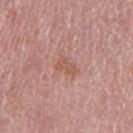Recorded during total-body skin imaging; not selected for excision or biopsy. An algorithmic analysis of the crop reported an average lesion color of about L≈56 a*≈23 b*≈26 (CIELAB), roughly 7 lightness units darker than nearby skin, and a lesion-to-skin contrast of about 5.5 (normalized; higher = more distinct). This is a white-light tile. A female patient, about 70 years old. On the left thigh. Cropped from a total-body skin-imaging series; the visible field is about 15 mm.